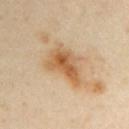This lesion was catalogued during total-body skin photography and was not selected for biopsy. From the arm. A 15 mm close-up extracted from a 3D total-body photography capture. The tile uses cross-polarized illumination. Approximately 5 mm at its widest. The patient is a male about 40 years old. Automated tile analysis of the lesion measured a nevus-likeness score of about 85/100 and a detector confidence of about 100 out of 100 that the crop contains a lesion.